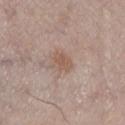Imaged during a routine full-body skin examination; the lesion was not biopsied and no histopathology is available. Automated tile analysis of the lesion measured a lesion area of about 4 mm², an outline eccentricity of about 0.7 (0 = round, 1 = elongated), and a shape-asymmetry score of about 0.2 (0 = symmetric). And it measured a border-irregularity rating of about 2/10, a color-variation rating of about 1.5/10, and peripheral color asymmetry of about 0.5. The analysis additionally found an automated nevus-likeness rating near 30 out of 100 and a detector confidence of about 100 out of 100 that the crop contains a lesion. The lesion's longest dimension is about 2.5 mm. This image is a 15 mm lesion crop taken from a total-body photograph. Imaged with white-light lighting. The lesion is located on the left lower leg. A male patient approximately 60 years of age.